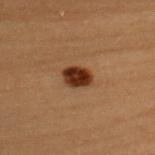Q: Is there a histopathology result?
A: imaged on a skin check; not biopsied